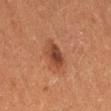notes: total-body-photography surveillance lesion; no biopsy | illumination: cross-polarized illumination | image: ~15 mm tile from a whole-body skin photo | site: the right thigh | subject: female, aged 48–52.